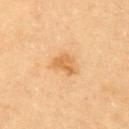Clinical impression: Part of a total-body skin-imaging series; this lesion was reviewed on a skin check and was not flagged for biopsy. Image and clinical context: From the back. Cropped from a total-body skin-imaging series; the visible field is about 15 mm. Imaged with cross-polarized lighting. Automated tile analysis of the lesion measured a footprint of about 5.5 mm², an eccentricity of roughly 0.65, and two-axis asymmetry of about 0.35. The patient is a male in their mid-80s or older.